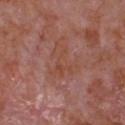Assessment:
The lesion was photographed on a routine skin check and not biopsied; there is no pathology result.
Acquisition and patient details:
The lesion's longest dimension is about 4.5 mm. An algorithmic analysis of the crop reported an automated nevus-likeness rating near 0 out of 100 and a lesion-detection confidence of about 100/100. A region of skin cropped from a whole-body photographic capture, roughly 15 mm wide. From the chest. Imaged with white-light lighting. A male patient, aged around 65.